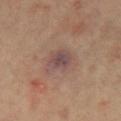Clinical impression: No biopsy was performed on this lesion — it was imaged during a full skin examination and was not determined to be concerning. Image and clinical context: The lesion's longest dimension is about 3.5 mm. The total-body-photography lesion software estimated an area of roughly 7.5 mm², an eccentricity of roughly 0.55, and a symmetry-axis asymmetry near 0.25. It also reported a mean CIELAB color near L≈46 a*≈17 b*≈20, a lesion–skin lightness drop of about 8, and a normalized lesion–skin contrast near 8. The software also gave border irregularity of about 2.5 on a 0–10 scale and internal color variation of about 3.5 on a 0–10 scale. The patient is a male about 65 years old. A close-up tile cropped from a whole-body skin photograph, about 15 mm across. The lesion is located on the chest. Imaged with cross-polarized lighting.